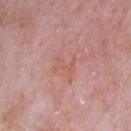Q: What are the patient's age and sex?
A: male, in their mid-60s
Q: What did automated image analysis measure?
A: a lesion color around L≈58 a*≈25 b*≈28 in CIELAB, a lesion–skin lightness drop of about 5, and a normalized border contrast of about 4.5; an automated nevus-likeness rating near 0 out of 100 and lesion-presence confidence of about 100/100
Q: Where on the body is the lesion?
A: the chest
Q: What is the imaging modality?
A: ~15 mm tile from a whole-body skin photo
Q: Illumination type?
A: white-light illumination